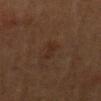<record>
<biopsy_status>not biopsied; imaged during a skin examination</biopsy_status>
<site>abdomen</site>
<patient>
  <sex>male</sex>
  <age_approx>55</age_approx>
</patient>
<image>
  <source>total-body photography crop</source>
  <field_of_view_mm>15</field_of_view_mm>
</image>
</record>